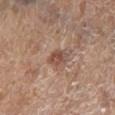Clinical impression:
Captured during whole-body skin photography for melanoma surveillance; the lesion was not biopsied.
Background:
The lesion is located on the leg. A 15 mm crop from a total-body photograph taken for skin-cancer surveillance. A female patient, approximately 85 years of age or older. This is a white-light tile.